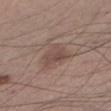workup — no biopsy performed (imaged during a skin exam); lesion size — ~3.5 mm (longest diameter); subject — male, about 55 years old; automated metrics — a lesion area of about 7 mm², an outline eccentricity of about 0.6 (0 = round, 1 = elongated), and two-axis asymmetry of about 0.4; imaging modality — ~15 mm crop, total-body skin-cancer survey; body site — the arm; illumination — white-light illumination.